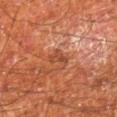Impression: Recorded during total-body skin imaging; not selected for excision or biopsy. Clinical summary: The lesion's longest dimension is about 2.5 mm. The subject is a male aged 63 to 67. From the right lower leg. Cropped from a total-body skin-imaging series; the visible field is about 15 mm. This is a cross-polarized tile.